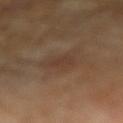Clinical impression:
The lesion was photographed on a routine skin check and not biopsied; there is no pathology result.
Background:
A male patient, approximately 85 years of age or older. Located on the right forearm. A 15 mm crop from a total-body photograph taken for skin-cancer surveillance. Captured under cross-polarized illumination. Automated tile analysis of the lesion measured a lesion area of about 3.5 mm², a shape eccentricity near 0.85, and a shape-asymmetry score of about 0.3 (0 = symmetric). Measured at roughly 2.5 mm in maximum diameter.